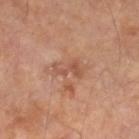Findings:
– workup — no biopsy performed (imaged during a skin exam)
– site — the right lower leg
– subject — male, in their mid-60s
– lesion diameter — about 3.5 mm
– tile lighting — cross-polarized illumination
– imaging modality — ~15 mm crop, total-body skin-cancer survey